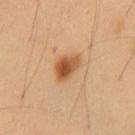workup: no biopsy performed (imaged during a skin exam) | image source: ~15 mm tile from a whole-body skin photo | site: the chest | TBP lesion metrics: a mean CIELAB color near L≈52 a*≈22 b*≈38 and a lesion-to-skin contrast of about 9.5 (normalized; higher = more distinct) | tile lighting: cross-polarized illumination | subject: male, about 55 years old.